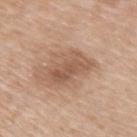The lesion was photographed on a routine skin check and not biopsied; there is no pathology result.